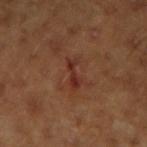Case summary:
- follow-up — catalogued during a skin exam; not biopsied
- lesion diameter — about 3.5 mm
- lighting — cross-polarized illumination
- image — total-body-photography crop, ~15 mm field of view
- subject — male, aged 58–62
- automated metrics — a lesion color around L≈30 a*≈23 b*≈26 in CIELAB, about 7 CIELAB-L* units darker than the surrounding skin, and a normalized lesion–skin contrast near 7; border irregularity of about 5.5 on a 0–10 scale, a within-lesion color-variation index near 1.5/10, and a peripheral color-asymmetry measure near 0.5
- location — the right forearm